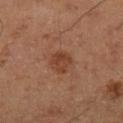Cropped from a whole-body photographic skin survey; the tile spans about 15 mm. A male subject, aged 58–62. About 3 mm across. The total-body-photography lesion software estimated a shape eccentricity near 0.55. It also reported roughly 8 lightness units darker than nearby skin and a lesion-to-skin contrast of about 7 (normalized; higher = more distinct). It also reported a within-lesion color-variation index near 3.5/10. And it measured a classifier nevus-likeness of about 40/100 and a lesion-detection confidence of about 100/100. The tile uses cross-polarized illumination. The lesion is on the left lower leg.